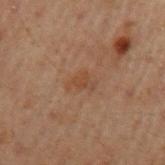Clinical impression: Captured during whole-body skin photography for melanoma surveillance; the lesion was not biopsied. Clinical summary: This is a cross-polarized tile. A lesion tile, about 15 mm wide, cut from a 3D total-body photograph. Measured at roughly 3 mm in maximum diameter. Located on the left upper arm. The total-body-photography lesion software estimated a lesion color around L≈34 a*≈16 b*≈25 in CIELAB, roughly 5 lightness units darker than nearby skin, and a lesion-to-skin contrast of about 5.5 (normalized; higher = more distinct). It also reported a nevus-likeness score of about 0/100 and lesion-presence confidence of about 100/100. A male subject in their 60s.